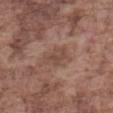Clinical impression: Part of a total-body skin-imaging series; this lesion was reviewed on a skin check and was not flagged for biopsy. Image and clinical context: An algorithmic analysis of the crop reported an area of roughly 5 mm², an eccentricity of roughly 0.75, and a symmetry-axis asymmetry near 0.35. It also reported a border-irregularity index near 4.5/10 and internal color variation of about 2.5 on a 0–10 scale. And it measured a classifier nevus-likeness of about 0/100 and a detector confidence of about 100 out of 100 that the crop contains a lesion. Located on the abdomen. Captured under white-light illumination. Approximately 3.5 mm at its widest. A 15 mm crop from a total-body photograph taken for skin-cancer surveillance. The patient is a male approximately 75 years of age.